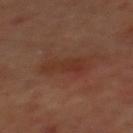{
  "biopsy_status": "not biopsied; imaged during a skin examination",
  "site": "mid back",
  "automated_metrics": {
    "cielab_L": 31,
    "cielab_a": 21,
    "cielab_b": 27,
    "vs_skin_darker_L": 5.0,
    "vs_skin_contrast_norm": 5.5,
    "border_irregularity_0_10": 4.0,
    "color_variation_0_10": 3.0,
    "peripheral_color_asymmetry": 1.0,
    "nevus_likeness_0_100": 0,
    "lesion_detection_confidence_0_100": 100
  },
  "lighting": "cross-polarized",
  "patient": {
    "sex": "male",
    "age_approx": 70
  },
  "image": {
    "source": "total-body photography crop",
    "field_of_view_mm": 15
  }
}